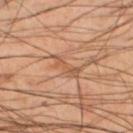Q: Is there a histopathology result?
A: no biopsy performed (imaged during a skin exam)
Q: What is the imaging modality?
A: ~15 mm tile from a whole-body skin photo
Q: What are the patient's age and sex?
A: male, aged around 50
Q: What is the anatomic site?
A: the left lower leg